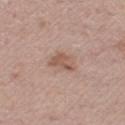Q: Was a biopsy performed?
A: total-body-photography surveillance lesion; no biopsy
Q: Illumination type?
A: white-light
Q: What is the anatomic site?
A: the left thigh
Q: Automated lesion metrics?
A: an eccentricity of roughly 0.7 and a shape-asymmetry score of about 0.35 (0 = symmetric); border irregularity of about 4 on a 0–10 scale, internal color variation of about 2.5 on a 0–10 scale, and peripheral color asymmetry of about 1; a classifier nevus-likeness of about 20/100 and a lesion-detection confidence of about 100/100
Q: How was this image acquired?
A: ~15 mm crop, total-body skin-cancer survey
Q: How large is the lesion?
A: ≈3.5 mm
Q: What are the patient's age and sex?
A: male, in their mid- to late 50s